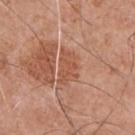Q: Lesion size?
A: ≈4 mm
Q: What did automated image analysis measure?
A: a mean CIELAB color near L≈54 a*≈25 b*≈32, about 8 CIELAB-L* units darker than the surrounding skin, and a normalized border contrast of about 6; border irregularity of about 4.5 on a 0–10 scale, a within-lesion color-variation index near 2.5/10, and radial color variation of about 1
Q: What kind of image is this?
A: total-body-photography crop, ~15 mm field of view
Q: What lighting was used for the tile?
A: white-light
Q: What is the anatomic site?
A: the chest
Q: Patient demographics?
A: male, about 55 years old